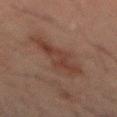biopsy_status: not biopsied; imaged during a skin examination
automated_metrics:
  area_mm2_approx: 17.0
  eccentricity: 0.9
  shape_asymmetry: 0.35
  border_irregularity_0_10: 5.0
  color_variation_0_10: 3.0
  peripheral_color_asymmetry: 1.0
patient:
  sex: male
  age_approx: 50
site: back
lighting: cross-polarized
image:
  source: total-body photography crop
  field_of_view_mm: 15